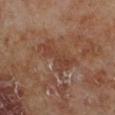No biopsy was performed on this lesion — it was imaged during a full skin examination and was not determined to be concerning.
About 5.5 mm across.
A close-up tile cropped from a whole-body skin photograph, about 15 mm across.
A male patient, aged 68–72.
On the leg.